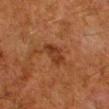Impression: Recorded during total-body skin imaging; not selected for excision or biopsy.